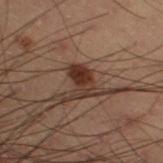Recorded during total-body skin imaging; not selected for excision or biopsy. The total-body-photography lesion software estimated an area of roughly 6.5 mm². The analysis additionally found a classifier nevus-likeness of about 95/100 and a lesion-detection confidence of about 100/100. From the leg. The patient is a male aged 53–57. A roughly 15 mm field-of-view crop from a total-body skin photograph. Approximately 3.5 mm at its widest. Imaged with cross-polarized lighting.